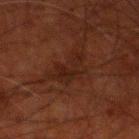notes = no biopsy performed (imaged during a skin exam)
diameter = ~5 mm (longest diameter)
lighting = cross-polarized
anatomic site = the leg
image source = ~15 mm tile from a whole-body skin photo
subject = male, roughly 80 years of age
image-analysis metrics = a lesion color around L≈17 a*≈17 b*≈20 in CIELAB and a lesion–skin lightness drop of about 4; a classifier nevus-likeness of about 0/100 and a lesion-detection confidence of about 95/100